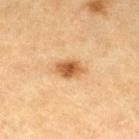<record>
  <biopsy_status>not biopsied; imaged during a skin examination</biopsy_status>
  <patient>
    <sex>female</sex>
    <age_approx>55</age_approx>
  </patient>
  <lighting>cross-polarized</lighting>
  <image>
    <source>total-body photography crop</source>
    <field_of_view_mm>15</field_of_view_mm>
  </image>
  <site>left lower leg</site>
  <lesion_size>
    <long_diameter_mm_approx>4.0</long_diameter_mm_approx>
  </lesion_size>
</record>